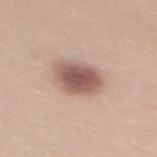| key | value |
|---|---|
| workup | no biopsy performed (imaged during a skin exam) |
| subject | female, aged approximately 35 |
| image | ~15 mm crop, total-body skin-cancer survey |
| location | the upper back |